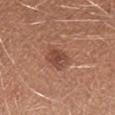{"patient": {"sex": "female", "age_approx": 25}, "lesion_size": {"long_diameter_mm_approx": 3.0}, "lighting": "white-light", "site": "right forearm", "image": {"source": "total-body photography crop", "field_of_view_mm": 15}, "automated_metrics": {"border_irregularity_0_10": 1.5, "color_variation_0_10": 4.0, "peripheral_color_asymmetry": 1.0, "nevus_likeness_0_100": 30}}